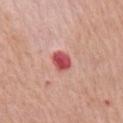This lesion was catalogued during total-body skin photography and was not selected for biopsy.
A 15 mm close-up extracted from a 3D total-body photography capture.
Measured at roughly 3 mm in maximum diameter.
Captured under white-light illumination.
The lesion is on the right upper arm.
The subject is a male aged approximately 65.